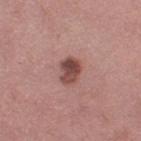follow-up = no biopsy performed (imaged during a skin exam) | location = the leg | lesion size = ≈3 mm | patient = female, in their 40s | image = ~15 mm tile from a whole-body skin photo | lighting = white-light.